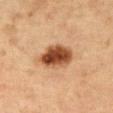| field | value |
|---|---|
| TBP lesion metrics | a classifier nevus-likeness of about 100/100 and lesion-presence confidence of about 100/100 |
| location | the chest |
| illumination | cross-polarized |
| subject | female, in their 60s |
| diameter | about 5 mm |
| imaging modality | ~15 mm crop, total-body skin-cancer survey |